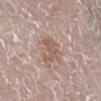Clinical impression:
This lesion was catalogued during total-body skin photography and was not selected for biopsy.
Background:
A male patient approximately 80 years of age. The lesion is on the right lower leg. Cropped from a total-body skin-imaging series; the visible field is about 15 mm. Captured under white-light illumination.